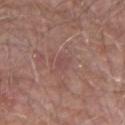biopsy status — no biopsy performed (imaged during a skin exam); subject — male, aged around 65; anatomic site — the left forearm; image source — total-body-photography crop, ~15 mm field of view.